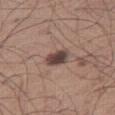Q: Was this lesion biopsied?
A: imaged on a skin check; not biopsied
Q: What is the lesion's diameter?
A: about 3 mm
Q: How was the tile lit?
A: white-light illumination
Q: Patient demographics?
A: male, about 65 years old
Q: Lesion location?
A: the right thigh
Q: What kind of image is this?
A: 15 mm crop, total-body photography